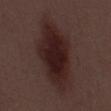site = the back
patient = male, roughly 55 years of age
diameter = about 11 mm
lighting = white-light illumination
image source = ~15 mm tile from a whole-body skin photo